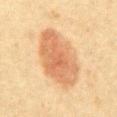Impression: Recorded during total-body skin imaging; not selected for excision or biopsy.